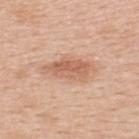Part of a total-body skin-imaging series; this lesion was reviewed on a skin check and was not flagged for biopsy. A male subject, approximately 50 years of age. On the back. A 15 mm close-up extracted from a 3D total-body photography capture. Captured under white-light illumination.